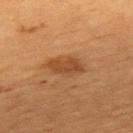No biopsy was performed on this lesion — it was imaged during a full skin examination and was not determined to be concerning. A close-up tile cropped from a whole-body skin photograph, about 15 mm across. The recorded lesion diameter is about 4 mm. On the upper back. A male patient, aged approximately 85 or older.